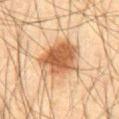The lesion-visualizer software estimated an area of roughly 20 mm², an eccentricity of roughly 0.5, and a shape-asymmetry score of about 0.2 (0 = symmetric). The analysis additionally found a border-irregularity rating of about 2.5/10. And it measured a nevus-likeness score of about 100/100 and a detector confidence of about 100 out of 100 that the crop contains a lesion. A male patient aged 63 to 67. Imaged with cross-polarized lighting. From the abdomen. A 15 mm close-up tile from a total-body photography series done for melanoma screening. Approximately 5.5 mm at its widest.